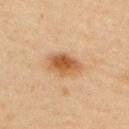<tbp_lesion>
  <biopsy_status>not biopsied; imaged during a skin examination</biopsy_status>
  <site>right upper arm</site>
  <automated_metrics>
    <area_mm2_approx>7.5</area_mm2_approx>
    <eccentricity>0.8</eccentricity>
    <shape_asymmetry>0.2</shape_asymmetry>
  </automated_metrics>
  <lesion_size>
    <long_diameter_mm_approx>4.0</long_diameter_mm_approx>
  </lesion_size>
  <image>
    <source>total-body photography crop</source>
    <field_of_view_mm>15</field_of_view_mm>
  </image>
  <patient>
    <sex>female</sex>
    <age_approx>45</age_approx>
  </patient>
</tbp_lesion>